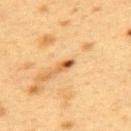notes: imaged on a skin check; not biopsied
image: ~15 mm tile from a whole-body skin photo
site: the upper back
patient: female, aged 38–42
lighting: cross-polarized illumination
lesion size: ~2.5 mm (longest diameter)